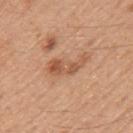follow-up = catalogued during a skin exam; not biopsied
lesion diameter = about 4.5 mm
acquisition = total-body-photography crop, ~15 mm field of view
anatomic site = the mid back
patient = male, roughly 50 years of age
lighting = white-light illumination